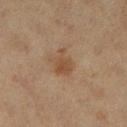| feature | finding |
|---|---|
| workup | total-body-photography surveillance lesion; no biopsy |
| patient | female, aged around 40 |
| anatomic site | the right lower leg |
| image | total-body-photography crop, ~15 mm field of view |